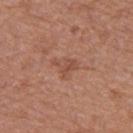<tbp_lesion>
<biopsy_status>not biopsied; imaged during a skin examination</biopsy_status>
<site>left thigh</site>
<image>
  <source>total-body photography crop</source>
  <field_of_view_mm>15</field_of_view_mm>
</image>
<patient>
  <sex>female</sex>
  <age_approx>40</age_approx>
</patient>
</tbp_lesion>